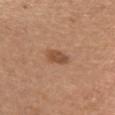| key | value |
|---|---|
| workup | imaged on a skin check; not biopsied |
| imaging modality | total-body-photography crop, ~15 mm field of view |
| patient | female, aged around 35 |
| site | the chest |